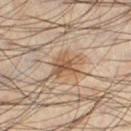Imaged during a routine full-body skin examination; the lesion was not biopsied and no histopathology is available. This is a cross-polarized tile. A male patient, in their mid- to late 40s. The lesion is located on the leg. Automated image analysis of the tile measured an eccentricity of roughly 0.6 and two-axis asymmetry of about 0.35. And it measured a lesion color around L≈53 a*≈17 b*≈31 in CIELAB. Measured at roughly 3.5 mm in maximum diameter. Cropped from a whole-body photographic skin survey; the tile spans about 15 mm.